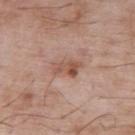biopsy status: imaged on a skin check; not biopsied | patient: male, roughly 65 years of age | body site: the upper back | image-analysis metrics: a lesion area of about 6.5 mm², an eccentricity of roughly 0.85, and two-axis asymmetry of about 0.25; an average lesion color of about L≈54 a*≈21 b*≈28 (CIELAB) and a lesion-to-skin contrast of about 7 (normalized; higher = more distinct); border irregularity of about 3 on a 0–10 scale, a color-variation rating of about 6/10, and peripheral color asymmetry of about 2 | acquisition: ~15 mm crop, total-body skin-cancer survey.